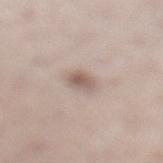Recorded during total-body skin imaging; not selected for excision or biopsy.
A female patient, approximately 50 years of age.
The lesion is on the left lower leg.
A 15 mm close-up extracted from a 3D total-body photography capture.
The tile uses white-light illumination.
Automated image analysis of the tile measured a footprint of about 3.5 mm², an eccentricity of roughly 0.75, and two-axis asymmetry of about 0.3.
Longest diameter approximately 2.5 mm.